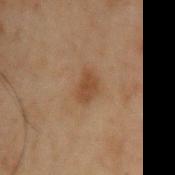Part of a total-body skin-imaging series; this lesion was reviewed on a skin check and was not flagged for biopsy. Located on the left upper arm. The tile uses cross-polarized illumination. Cropped from a whole-body photographic skin survey; the tile spans about 15 mm. A male patient, aged around 70. Automated tile analysis of the lesion measured a symmetry-axis asymmetry near 0.25.